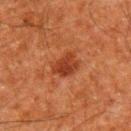Impression:
Recorded during total-body skin imaging; not selected for excision or biopsy.
Clinical summary:
On the left upper arm. An algorithmic analysis of the crop reported a mean CIELAB color near L≈31 a*≈26 b*≈31 and roughly 9 lightness units darker than nearby skin. The software also gave border irregularity of about 3.5 on a 0–10 scale, internal color variation of about 2 on a 0–10 scale, and peripheral color asymmetry of about 0.5. A lesion tile, about 15 mm wide, cut from a 3D total-body photograph. Longest diameter approximately 3.5 mm. Imaged with cross-polarized lighting. A male patient aged around 60.